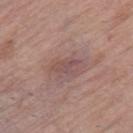biopsy_status: not biopsied; imaged during a skin examination
image:
  source: total-body photography crop
  field_of_view_mm: 15
lesion_size:
  long_diameter_mm_approx: 3.0
patient:
  sex: female
  age_approx: 65
lighting: white-light
site: right thigh